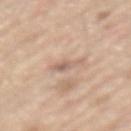biopsy_status: not biopsied; imaged during a skin examination
site: back
image:
  source: total-body photography crop
  field_of_view_mm: 15
patient:
  sex: male
  age_approx: 70
lesion_size:
  long_diameter_mm_approx: 3.0
lighting: white-light